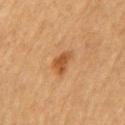A region of skin cropped from a whole-body photographic capture, roughly 15 mm wide.
The patient is a male aged around 65.
From the chest.